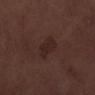Recorded during total-body skin imaging; not selected for excision or biopsy.
On the left lower leg.
The tile uses white-light illumination.
The patient is a male approximately 70 years of age.
About 3 mm across.
Cropped from a whole-body photographic skin survey; the tile spans about 15 mm.